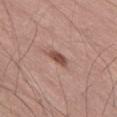notes = imaged on a skin check; not biopsied | lighting = white-light illumination | image-analysis metrics = a footprint of about 3.5 mm² and a shape eccentricity near 0.85; an average lesion color of about L≈49 a*≈22 b*≈26 (CIELAB) and about 13 CIELAB-L* units darker than the surrounding skin; a color-variation rating of about 2.5/10 | patient = male, in their mid- to late 50s | acquisition = 15 mm crop, total-body photography.